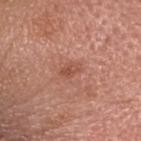The total-body-photography lesion software estimated roughly 8 lightness units darker than nearby skin. The analysis additionally found a border-irregularity index near 2/10, internal color variation of about 3 on a 0–10 scale, and a peripheral color-asymmetry measure near 1.
The patient is a male approximately 60 years of age.
About 2.5 mm across.
Located on the head or neck.
A 15 mm close-up extracted from a 3D total-body photography capture.
This is a white-light tile.